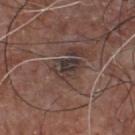Captured during whole-body skin photography for melanoma surveillance; the lesion was not biopsied.
Longest diameter approximately 3.5 mm.
From the chest.
A region of skin cropped from a whole-body photographic capture, roughly 15 mm wide.
The subject is a male roughly 75 years of age.
Imaged with white-light lighting.